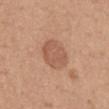follow-up: total-body-photography surveillance lesion; no biopsy
acquisition: total-body-photography crop, ~15 mm field of view
diameter: ~4 mm (longest diameter)
subject: female, about 35 years old
anatomic site: the mid back
automated metrics: an area of roughly 11 mm², an eccentricity of roughly 0.45, and two-axis asymmetry of about 0.1